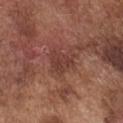{
  "biopsy_status": "not biopsied; imaged during a skin examination",
  "lighting": "white-light",
  "lesion_size": {
    "long_diameter_mm_approx": 3.0
  },
  "site": "chest",
  "patient": {
    "sex": "male",
    "age_approx": 75
  },
  "automated_metrics": {
    "border_irregularity_0_10": 4.0,
    "color_variation_0_10": 2.0
  },
  "image": {
    "source": "total-body photography crop",
    "field_of_view_mm": 15
  }
}